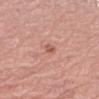site: the right upper arm
image source: ~15 mm crop, total-body skin-cancer survey
patient: female, aged 63–67